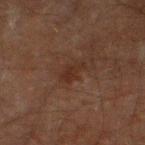Findings:
- biopsy status · no biopsy performed (imaged during a skin exam)
- image-analysis metrics · a shape eccentricity near 0.9; an average lesion color of about L≈21 a*≈15 b*≈20 (CIELAB), about 5 CIELAB-L* units darker than the surrounding skin, and a lesion-to-skin contrast of about 6.5 (normalized; higher = more distinct); a within-lesion color-variation index near 0.5/10; a nevus-likeness score of about 0/100
- subject · male, in their 60s
- acquisition · total-body-photography crop, ~15 mm field of view
- body site · the left thigh